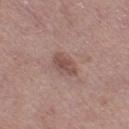A female patient aged 48 to 52. A 15 mm close-up extracted from a 3D total-body photography capture. The lesion is located on the right thigh.